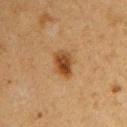workup — total-body-photography surveillance lesion; no biopsy | TBP lesion metrics — a lesion color around L≈40 a*≈19 b*≈34 in CIELAB and a lesion-to-skin contrast of about 9 (normalized; higher = more distinct); a border-irregularity rating of about 2.5/10, a within-lesion color-variation index near 5.5/10, and peripheral color asymmetry of about 1.5; a nevus-likeness score of about 95/100 and a detector confidence of about 100 out of 100 that the crop contains a lesion | illumination — cross-polarized | subject — female, roughly 40 years of age | body site — the arm | image source — total-body-photography crop, ~15 mm field of view | lesion size — ~3.5 mm (longest diameter).